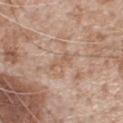Findings:
– subject: male, in their mid- to late 60s
– location: the chest
– acquisition: ~15 mm tile from a whole-body skin photo
– diameter: ≈3 mm